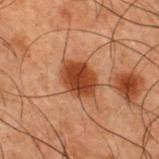Q: Was a biopsy performed?
A: catalogued during a skin exam; not biopsied
Q: Patient demographics?
A: male, roughly 50 years of age
Q: Lesion location?
A: the upper back
Q: What kind of image is this?
A: ~15 mm tile from a whole-body skin photo
Q: How large is the lesion?
A: about 4 mm